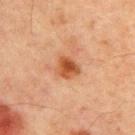An algorithmic analysis of the crop reported a border-irregularity rating of about 2.5/10, a color-variation rating of about 3.5/10, and radial color variation of about 1. It also reported a lesion-detection confidence of about 100/100.
Cropped from a total-body skin-imaging series; the visible field is about 15 mm.
The patient is a male approximately 65 years of age.
This is a cross-polarized tile.
Longest diameter approximately 2.5 mm.
Located on the chest.